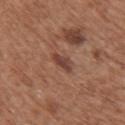The lesion was photographed on a routine skin check and not biopsied; there is no pathology result.